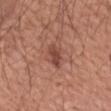This lesion was catalogued during total-body skin photography and was not selected for biopsy. A 15 mm close-up tile from a total-body photography series done for melanoma screening. The tile uses white-light illumination. About 3 mm across. Automated tile analysis of the lesion measured a mean CIELAB color near L≈46 a*≈24 b*≈27, a lesion–skin lightness drop of about 10, and a normalized border contrast of about 7.5. The analysis additionally found border irregularity of about 2 on a 0–10 scale and internal color variation of about 3.5 on a 0–10 scale. From the left forearm. A male subject roughly 50 years of age.